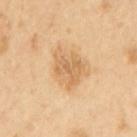Case summary:
* notes — total-body-photography surveillance lesion; no biopsy
* body site — the upper back
* lesion size — ~4.5 mm (longest diameter)
* patient — female, roughly 55 years of age
* image source — total-body-photography crop, ~15 mm field of view